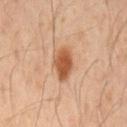{"biopsy_status": "not biopsied; imaged during a skin examination", "site": "mid back", "patient": {"sex": "male", "age_approx": 50}, "image": {"source": "total-body photography crop", "field_of_view_mm": 15}, "lesion_size": {"long_diameter_mm_approx": 4.0}}